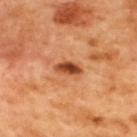No biopsy was performed on this lesion — it was imaged during a full skin examination and was not determined to be concerning. Approximately 3 mm at its widest. A male subject aged 48–52. The lesion is on the upper back. A 15 mm crop from a total-body photograph taken for skin-cancer surveillance. This is a cross-polarized tile.